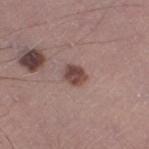A close-up tile cropped from a whole-body skin photograph, about 15 mm across. The lesion is on the right thigh. Imaged with white-light lighting. A male subject aged 43–47.